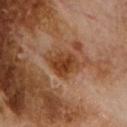Assessment: Imaged during a routine full-body skin examination; the lesion was not biopsied and no histopathology is available. Image and clinical context: Longest diameter approximately 3.5 mm. From the chest. A male patient aged approximately 70. Automated image analysis of the tile measured an area of roughly 9 mm², an eccentricity of roughly 0.6, and two-axis asymmetry of about 0.25. And it measured an automated nevus-likeness rating near 15 out of 100 and a detector confidence of about 100 out of 100 that the crop contains a lesion. A 15 mm close-up extracted from a 3D total-body photography capture.